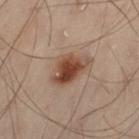workup: no biopsy performed (imaged during a skin exam) | tile lighting: cross-polarized illumination | TBP lesion metrics: an area of roughly 10 mm², an eccentricity of roughly 0.7, and a shape-asymmetry score of about 0.25 (0 = symmetric); an automated nevus-likeness rating near 100 out of 100 and lesion-presence confidence of about 100/100 | anatomic site: the abdomen | subject: male, roughly 50 years of age | image: total-body-photography crop, ~15 mm field of view.